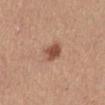{
  "biopsy_status": "not biopsied; imaged during a skin examination",
  "patient": {
    "sex": "female",
    "age_approx": 55
  },
  "lighting": "white-light",
  "site": "abdomen",
  "image": {
    "source": "total-body photography crop",
    "field_of_view_mm": 15
  }
}